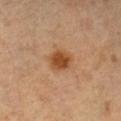Q: Is there a histopathology result?
A: imaged on a skin check; not biopsied
Q: What is the imaging modality?
A: total-body-photography crop, ~15 mm field of view
Q: Patient demographics?
A: female, approximately 60 years of age
Q: Where on the body is the lesion?
A: the chest